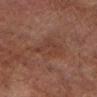{
  "biopsy_status": "not biopsied; imaged during a skin examination",
  "automated_metrics": {
    "shape_asymmetry": 0.35,
    "border_irregularity_0_10": 4.0,
    "color_variation_0_10": 3.0,
    "peripheral_color_asymmetry": 1.0
  },
  "site": "left lower leg",
  "lighting": "cross-polarized",
  "image": {
    "source": "total-body photography crop",
    "field_of_view_mm": 15
  },
  "lesion_size": {
    "long_diameter_mm_approx": 4.5
  },
  "patient": {
    "sex": "male",
    "age_approx": 75
  }
}